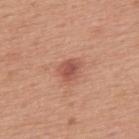A male patient aged around 75. This is a white-light tile. The lesion is located on the upper back. A 15 mm close-up extracted from a 3D total-body photography capture. The total-body-photography lesion software estimated an average lesion color of about L≈53 a*≈27 b*≈29 (CIELAB), about 11 CIELAB-L* units darker than the surrounding skin, and a lesion-to-skin contrast of about 7.5 (normalized; higher = more distinct). The software also gave a classifier nevus-likeness of about 70/100. The recorded lesion diameter is about 2.5 mm.